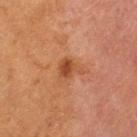biopsy status=total-body-photography surveillance lesion; no biopsy | patient=female, aged 48 to 52 | lighting=cross-polarized illumination | diameter=≈3 mm | site=the left thigh | image=~15 mm tile from a whole-body skin photo.